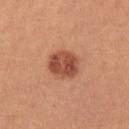workup = imaged on a skin check; not biopsied
automated metrics = a border-irregularity rating of about 1.5/10, a within-lesion color-variation index near 5/10, and radial color variation of about 2; a classifier nevus-likeness of about 85/100
lesion diameter = about 4 mm
tile lighting = white-light
patient = female, aged approximately 25
image = 15 mm crop, total-body photography
site = the left upper arm